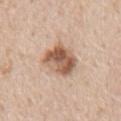notes — no biopsy performed (imaged during a skin exam) | subject — male, roughly 65 years of age | automated lesion analysis — a border-irregularity rating of about 2.5/10, internal color variation of about 7.5 on a 0–10 scale, and a peripheral color-asymmetry measure near 2.5; a classifier nevus-likeness of about 80/100 and a detector confidence of about 100 out of 100 that the crop contains a lesion | lesion diameter — ≈4.5 mm | illumination — white-light illumination | image source — 15 mm crop, total-body photography | site — the mid back.